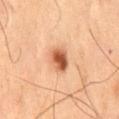No biopsy was performed on this lesion — it was imaged during a full skin examination and was not determined to be concerning. Automated image analysis of the tile measured an area of roughly 6 mm², an eccentricity of roughly 0.7, and a symmetry-axis asymmetry near 0.15. The analysis additionally found an average lesion color of about L≈58 a*≈27 b*≈38 (CIELAB), about 17 CIELAB-L* units darker than the surrounding skin, and a normalized border contrast of about 10.5. It also reported a border-irregularity index near 1.5/10, a color-variation rating of about 6/10, and a peripheral color-asymmetry measure near 2. It also reported a classifier nevus-likeness of about 95/100. A male patient, in their 60s. From the mid back. Imaged with cross-polarized lighting. This image is a 15 mm lesion crop taken from a total-body photograph.